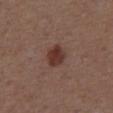This lesion was catalogued during total-body skin photography and was not selected for biopsy. Cropped from a total-body skin-imaging series; the visible field is about 15 mm. The tile uses white-light illumination. A male subject, aged approximately 55. The lesion is located on the abdomen.